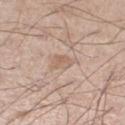Part of a total-body skin-imaging series; this lesion was reviewed on a skin check and was not flagged for biopsy. The tile uses white-light illumination. The recorded lesion diameter is about 3 mm. On the right thigh. A roughly 15 mm field-of-view crop from a total-body skin photograph. An algorithmic analysis of the crop reported an outline eccentricity of about 0.75 (0 = round, 1 = elongated) and a symmetry-axis asymmetry near 0.35. It also reported about 7 CIELAB-L* units darker than the surrounding skin and a normalized lesion–skin contrast near 5.5. And it measured a within-lesion color-variation index near 2/10. The subject is a male aged around 55.